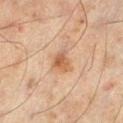Imaged during a routine full-body skin examination; the lesion was not biopsied and no histopathology is available. About 2.5 mm across. The lesion is located on the leg. The patient is a male aged around 45. Cropped from a total-body skin-imaging series; the visible field is about 15 mm.